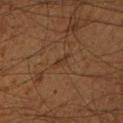Notes:
- workup · no biopsy performed (imaged during a skin exam)
- tile lighting · cross-polarized
- acquisition · total-body-photography crop, ~15 mm field of view
- location · the left lower leg
- subject · male, aged 58–62
- diameter · about 3 mm